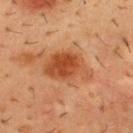image-analysis metrics — a shape eccentricity near 0.75 and a shape-asymmetry score of about 0.25 (0 = symmetric); about 10 CIELAB-L* units darker than the surrounding skin and a normalized border contrast of about 8.5 | tile lighting — cross-polarized illumination | diameter — ~5.5 mm (longest diameter) | subject — male, aged approximately 55 | site — the chest | image source — ~15 mm crop, total-body skin-cancer survey.